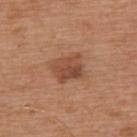Findings:
• illumination — white-light illumination
• TBP lesion metrics — a shape eccentricity near 0.6 and a symmetry-axis asymmetry near 0.2
• site — the upper back
• size — ~3.5 mm (longest diameter)
• patient — male, aged around 65
• imaging modality — total-body-photography crop, ~15 mm field of view